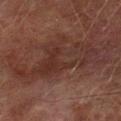<record>
<biopsy_status>not biopsied; imaged during a skin examination</biopsy_status>
<automated_metrics>
  <cielab_L>25</cielab_L>
  <cielab_a>17</cielab_a>
  <cielab_b>19</cielab_b>
  <vs_skin_darker_L>6.0</vs_skin_darker_L>
  <vs_skin_contrast_norm>6.5</vs_skin_contrast_norm>
  <nevus_likeness_0_100>0</nevus_likeness_0_100>
  <lesion_detection_confidence_0_100>90</lesion_detection_confidence_0_100>
</automated_metrics>
<lighting>cross-polarized</lighting>
<image>
  <source>total-body photography crop</source>
  <field_of_view_mm>15</field_of_view_mm>
</image>
<patient>
  <sex>male</sex>
  <age_approx>75</age_approx>
</patient>
<site>left lower leg</site>
</record>